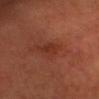<lesion>
<biopsy_status>not biopsied; imaged during a skin examination</biopsy_status>
<lighting>cross-polarized</lighting>
<image>
  <source>total-body photography crop</source>
  <field_of_view_mm>15</field_of_view_mm>
</image>
<site>head or neck</site>
<lesion_size>
  <long_diameter_mm_approx>3.5</long_diameter_mm_approx>
</lesion_size>
<automated_metrics>
  <cielab_L>30</cielab_L>
  <cielab_a>25</cielab_a>
  <cielab_b>28</cielab_b>
  <vs_skin_darker_L>6.0</vs_skin_darker_L>
  <vs_skin_contrast_norm>6.0</vs_skin_contrast_norm>
  <border_irregularity_0_10>4.0</border_irregularity_0_10>
  <color_variation_0_10>1.5</color_variation_0_10>
  <peripheral_color_asymmetry>0.5</peripheral_color_asymmetry>
  <nevus_likeness_0_100>0</nevus_likeness_0_100>
  <lesion_detection_confidence_0_100>100</lesion_detection_confidence_0_100>
</automated_metrics>
<patient>
  <sex>male</sex>
  <age_approx>60</age_approx>
</patient>
</lesion>